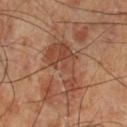| feature | finding |
|---|---|
| workup | no biopsy performed (imaged during a skin exam) |
| lesion size | ≈7 mm |
| site | the left lower leg |
| lighting | cross-polarized |
| acquisition | ~15 mm tile from a whole-body skin photo |
| patient | male, about 70 years old |